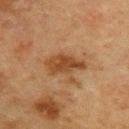biopsy status: no biopsy performed (imaged during a skin exam)
acquisition: 15 mm crop, total-body photography
location: the upper back
subject: female, in their mid- to late 50s
image-analysis metrics: an area of roughly 8.5 mm², an outline eccentricity of about 0.85 (0 = round, 1 = elongated), and a symmetry-axis asymmetry near 0.3; a border-irregularity index near 3.5/10 and internal color variation of about 3 on a 0–10 scale
tile lighting: cross-polarized illumination
lesion size: about 4.5 mm